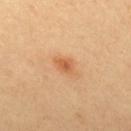A lesion tile, about 15 mm wide, cut from a 3D total-body photograph. The subject is a female in their 40s. The total-body-photography lesion software estimated an area of roughly 3.5 mm² and an eccentricity of roughly 0.75. The analysis additionally found a within-lesion color-variation index near 2/10 and a peripheral color-asymmetry measure near 0.5. The analysis additionally found a nevus-likeness score of about 85/100 and a detector confidence of about 100 out of 100 that the crop contains a lesion. On the upper back. About 2.5 mm across.